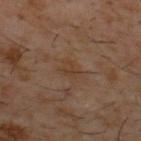{"biopsy_status": "not biopsied; imaged during a skin examination", "lighting": "cross-polarized", "site": "upper back", "lesion_size": {"long_diameter_mm_approx": 2.5}, "patient": {"sex": "male", "age_approx": 60}, "image": {"source": "total-body photography crop", "field_of_view_mm": 15}}